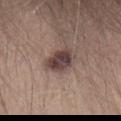- biopsy status — imaged on a skin check; not biopsied
- lighting — white-light illumination
- location — the lower back
- image — 15 mm crop, total-body photography
- subject — male, aged 23–27